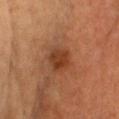Clinical impression:
Captured during whole-body skin photography for melanoma surveillance; the lesion was not biopsied.
Image and clinical context:
A female patient roughly 55 years of age. Captured under cross-polarized illumination. The total-body-photography lesion software estimated a mean CIELAB color near L≈33 a*≈22 b*≈30 and roughly 8 lightness units darker than nearby skin. It also reported a border-irregularity rating of about 1.5/10. And it measured an automated nevus-likeness rating near 90 out of 100 and a detector confidence of about 100 out of 100 that the crop contains a lesion. About 3 mm across. From the chest. A 15 mm close-up tile from a total-body photography series done for melanoma screening.